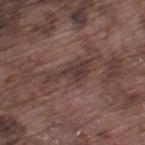workup: catalogued during a skin exam; not biopsied | diameter: ≈5.5 mm | automated lesion analysis: a lesion color around L≈36 a*≈17 b*≈19 in CIELAB and a lesion–skin lightness drop of about 8; a classifier nevus-likeness of about 0/100 | acquisition: 15 mm crop, total-body photography | patient: male, in their mid- to late 70s | location: the left thigh | lighting: white-light illumination.